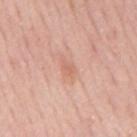Clinical impression:
Part of a total-body skin-imaging series; this lesion was reviewed on a skin check and was not flagged for biopsy.
Image and clinical context:
The tile uses white-light illumination. On the mid back. The patient is a male about 60 years old. Automated tile analysis of the lesion measured a lesion area of about 3.5 mm² and an outline eccentricity of about 0.8 (0 = round, 1 = elongated). The software also gave internal color variation of about 2 on a 0–10 scale and a peripheral color-asymmetry measure near 1. A lesion tile, about 15 mm wide, cut from a 3D total-body photograph.